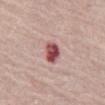Assessment: This lesion was catalogued during total-body skin photography and was not selected for biopsy. Background: The subject is a female roughly 65 years of age. A close-up tile cropped from a whole-body skin photograph, about 15 mm across. The lesion is on the abdomen. Captured under white-light illumination.